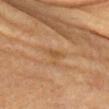Notes:
• notes: total-body-photography surveillance lesion; no biopsy
• image source: ~15 mm tile from a whole-body skin photo
• lesion diameter: about 3 mm
• subject: female, approximately 70 years of age
• location: the head or neck